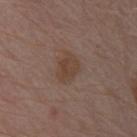notes = no biopsy performed (imaged during a skin exam) | image = ~15 mm tile from a whole-body skin photo | patient = male, roughly 80 years of age | anatomic site = the front of the torso | tile lighting = white-light | lesion diameter = about 3.5 mm.